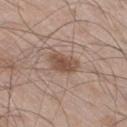Clinical impression:
Part of a total-body skin-imaging series; this lesion was reviewed on a skin check and was not flagged for biopsy.
Background:
A male patient, roughly 60 years of age. The tile uses white-light illumination. The lesion is located on the leg. A region of skin cropped from a whole-body photographic capture, roughly 15 mm wide. The lesion's longest dimension is about 3.5 mm.